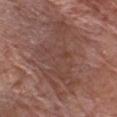Q: What lighting was used for the tile?
A: white-light
Q: What kind of image is this?
A: ~15 mm crop, total-body skin-cancer survey
Q: What did automated image analysis measure?
A: a mean CIELAB color near L≈43 a*≈20 b*≈24, roughly 7 lightness units darker than nearby skin, and a normalized border contrast of about 5.5; border irregularity of about 8.5 on a 0–10 scale and a color-variation rating of about 3/10; a nevus-likeness score of about 0/100 and a lesion-detection confidence of about 85/100
Q: What is the anatomic site?
A: the chest
Q: What are the patient's age and sex?
A: male, in their 80s